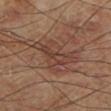Impression:
The lesion was tiled from a total-body skin photograph and was not biopsied.
Context:
Located on the left lower leg. A male subject aged approximately 70. Cropped from a total-body skin-imaging series; the visible field is about 15 mm. About 7 mm across. An algorithmic analysis of the crop reported a lesion–skin lightness drop of about 7 and a lesion-to-skin contrast of about 6.5 (normalized; higher = more distinct). The software also gave a border-irregularity rating of about 7/10, a color-variation rating of about 4.5/10, and peripheral color asymmetry of about 1.5.